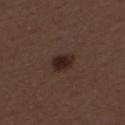A female subject aged approximately 50.
Located on the mid back.
Imaged with white-light lighting.
Measured at roughly 3 mm in maximum diameter.
Cropped from a whole-body photographic skin survey; the tile spans about 15 mm.